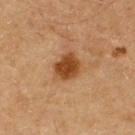| feature | finding |
|---|---|
| workup | total-body-photography surveillance lesion; no biopsy |
| size | ≈3.5 mm |
| image source | ~15 mm tile from a whole-body skin photo |
| subject | male, aged 58–62 |